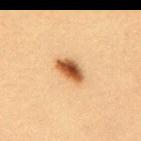workup = imaged on a skin check; not biopsied
subject = female, approximately 30 years of age
body site = the upper back
acquisition = ~15 mm tile from a whole-body skin photo
size = about 4 mm
automated lesion analysis = a mean CIELAB color near L≈53 a*≈22 b*≈39, about 17 CIELAB-L* units darker than the surrounding skin, and a normalized border contrast of about 11
lighting = cross-polarized illumination